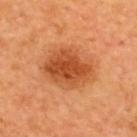Captured during whole-body skin photography for melanoma surveillance; the lesion was not biopsied.
Cropped from a total-body skin-imaging series; the visible field is about 15 mm.
A male patient, approximately 65 years of age.
The lesion is on the back.